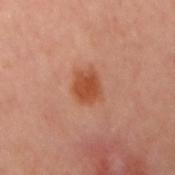Recorded during total-body skin imaging; not selected for excision or biopsy. The lesion is located on the left arm. Approximately 3.5 mm at its widest. The tile uses cross-polarized illumination. Automated image analysis of the tile measured a footprint of about 7.5 mm² and an eccentricity of roughly 0.55. The analysis additionally found a lesion color around L≈43 a*≈25 b*≈31 in CIELAB. The subject is a female in their 60s. A roughly 15 mm field-of-view crop from a total-body skin photograph.